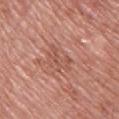Notes:
- workup · no biopsy performed (imaged during a skin exam)
- patient · male, aged 48–52
- illumination · white-light
- lesion diameter · about 4.5 mm
- automated lesion analysis · a lesion area of about 6.5 mm², an outline eccentricity of about 0.9 (0 = round, 1 = elongated), and a symmetry-axis asymmetry near 0.45; a mean CIELAB color near L≈54 a*≈25 b*≈28, about 7 CIELAB-L* units darker than the surrounding skin, and a normalized lesion–skin contrast near 5; border irregularity of about 6.5 on a 0–10 scale, a within-lesion color-variation index near 2/10, and a peripheral color-asymmetry measure near 0.5; an automated nevus-likeness rating near 0 out of 100
- acquisition · ~15 mm tile from a whole-body skin photo
- body site · the upper back